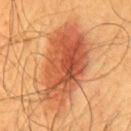Recorded during total-body skin imaging; not selected for excision or biopsy.
A close-up tile cropped from a whole-body skin photograph, about 15 mm across.
On the chest.
Imaged with cross-polarized lighting.
A male subject, in their 60s.
The total-body-photography lesion software estimated an eccentricity of roughly 0.85 and a symmetry-axis asymmetry near 0.2. The analysis additionally found an average lesion color of about L≈51 a*≈29 b*≈37 (CIELAB). The analysis additionally found a border-irregularity rating of about 3.5/10 and radial color variation of about 2. The software also gave an automated nevus-likeness rating near 100 out of 100 and lesion-presence confidence of about 100/100.